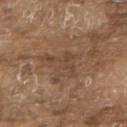Imaged during a routine full-body skin examination; the lesion was not biopsied and no histopathology is available.
An algorithmic analysis of the crop reported a footprint of about 7 mm², a shape eccentricity near 0.8, and a shape-asymmetry score of about 0.65 (0 = symmetric). It also reported a mean CIELAB color near L≈44 a*≈17 b*≈28, roughly 6 lightness units darker than nearby skin, and a lesion-to-skin contrast of about 5.5 (normalized; higher = more distinct). And it measured a border-irregularity rating of about 8.5/10, internal color variation of about 2.5 on a 0–10 scale, and a peripheral color-asymmetry measure near 1. The analysis additionally found an automated nevus-likeness rating near 0 out of 100 and a detector confidence of about 90 out of 100 that the crop contains a lesion.
A male subject aged around 80.
About 4.5 mm across.
Imaged with white-light lighting.
On the upper back.
A region of skin cropped from a whole-body photographic capture, roughly 15 mm wide.